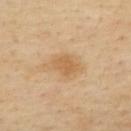follow-up — total-body-photography surveillance lesion; no biopsy
patient — female, in their mid-40s
body site — the back
illumination — cross-polarized
image — ~15 mm crop, total-body skin-cancer survey
automated metrics — a lesion area of about 9 mm², an eccentricity of roughly 0.7, and two-axis asymmetry of about 0.25; an average lesion color of about L≈62 a*≈17 b*≈39 (CIELAB) and a lesion-to-skin contrast of about 6 (normalized; higher = more distinct); border irregularity of about 3 on a 0–10 scale, internal color variation of about 2 on a 0–10 scale, and a peripheral color-asymmetry measure near 0.5